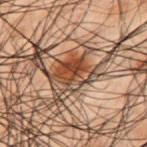Case summary:
* biopsy status · imaged on a skin check; not biopsied